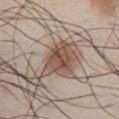Q: Is there a histopathology result?
A: total-body-photography surveillance lesion; no biopsy
Q: What is the imaging modality?
A: ~15 mm crop, total-body skin-cancer survey
Q: Automated lesion metrics?
A: a mean CIELAB color near L≈50 a*≈17 b*≈26, roughly 13 lightness units darker than nearby skin, and a normalized border contrast of about 9; a color-variation rating of about 5.5/10 and a peripheral color-asymmetry measure near 2; a classifier nevus-likeness of about 90/100 and lesion-presence confidence of about 100/100
Q: What lighting was used for the tile?
A: white-light
Q: How large is the lesion?
A: ~5.5 mm (longest diameter)
Q: Where on the body is the lesion?
A: the chest
Q: Patient demographics?
A: male, in their mid- to late 40s